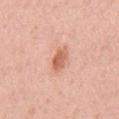This lesion was catalogued during total-body skin photography and was not selected for biopsy.
A male patient aged 53 to 57.
An algorithmic analysis of the crop reported a lesion color around L≈63 a*≈26 b*≈33 in CIELAB and a normalized lesion–skin contrast near 8. The software also gave border irregularity of about 2.5 on a 0–10 scale and a peripheral color-asymmetry measure near 0.5.
On the front of the torso.
The tile uses white-light illumination.
A 15 mm close-up extracted from a 3D total-body photography capture.
Approximately 3 mm at its widest.